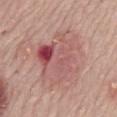  biopsy_status: not biopsied; imaged during a skin examination
  site: back
  image:
    source: total-body photography crop
    field_of_view_mm: 15
  patient:
    sex: male
    age_approx: 70
  automated_metrics:
    border_irregularity_0_10: 4.0
    color_variation_0_10: 10.0
    peripheral_color_asymmetry: 4.0